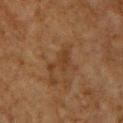{
  "biopsy_status": "not biopsied; imaged during a skin examination",
  "patient": {
    "sex": "male",
    "age_approx": 60
  },
  "image": {
    "source": "total-body photography crop",
    "field_of_view_mm": 15
  },
  "automated_metrics": {
    "eccentricity": 0.8,
    "shape_asymmetry": 0.6,
    "border_irregularity_0_10": 6.5,
    "color_variation_0_10": 1.5
  },
  "lesion_size": {
    "long_diameter_mm_approx": 4.0
  },
  "site": "chest",
  "lighting": "cross-polarized"
}